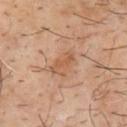| key | value |
|---|---|
| biopsy status | no biopsy performed (imaged during a skin exam) |
| automated lesion analysis | an eccentricity of roughly 0.9 and a shape-asymmetry score of about 0.35 (0 = symmetric); a lesion–skin lightness drop of about 7 and a normalized lesion–skin contrast near 6; a border-irregularity rating of about 4.5/10 and internal color variation of about 0 on a 0–10 scale; a detector confidence of about 100 out of 100 that the crop contains a lesion |
| anatomic site | the upper back |
| diameter | ~3.5 mm (longest diameter) |
| patient | male, in their mid-60s |
| image source | ~15 mm crop, total-body skin-cancer survey |
| tile lighting | cross-polarized illumination |